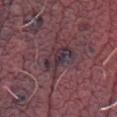Clinical impression:
The lesion was tiled from a total-body skin photograph and was not biopsied.
Acquisition and patient details:
Approximately 4.5 mm at its widest. Captured under white-light illumination. A male subject about 70 years old. The lesion is located on the left thigh. A 15 mm close-up tile from a total-body photography series done for melanoma screening.